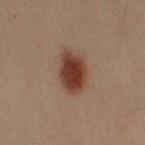The lesion was tiled from a total-body skin photograph and was not biopsied. Cropped from a whole-body photographic skin survey; the tile spans about 15 mm. On the arm. The patient is a male aged around 50. The tile uses cross-polarized illumination. About 5 mm across.